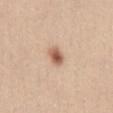Notes:
– follow-up: no biopsy performed (imaged during a skin exam)
– patient: female, in their mid-20s
– anatomic site: the abdomen
– acquisition: ~15 mm crop, total-body skin-cancer survey